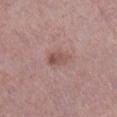workup = total-body-photography surveillance lesion; no biopsy
anatomic site = the right lower leg
subject = female, aged approximately 40
acquisition = 15 mm crop, total-body photography
size = ≈3 mm
illumination = white-light